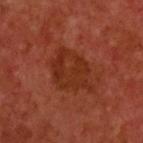biopsy status: catalogued during a skin exam; not biopsied | tile lighting: cross-polarized | body site: the upper back | imaging modality: 15 mm crop, total-body photography | TBP lesion metrics: an outline eccentricity of about 0.65 (0 = round, 1 = elongated) and a symmetry-axis asymmetry near 0.25; a lesion–skin lightness drop of about 6; a border-irregularity index near 3/10, a color-variation rating of about 3/10, and peripheral color asymmetry of about 1; an automated nevus-likeness rating near 0 out of 100 and a lesion-detection confidence of about 100/100 | patient: male, aged around 60.